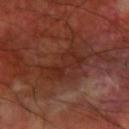The lesion was tiled from a total-body skin photograph and was not biopsied. A lesion tile, about 15 mm wide, cut from a 3D total-body photograph. On the right forearm. The subject is a male approximately 60 years of age.